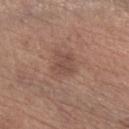A lesion tile, about 15 mm wide, cut from a 3D total-body photograph. The tile uses white-light illumination. The lesion is located on the left forearm. The subject is a male aged around 65.